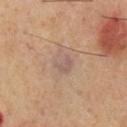| key | value |
|---|---|
| follow-up | imaged on a skin check; not biopsied |
| anatomic site | the chest |
| TBP lesion metrics | a footprint of about 4.5 mm², a shape eccentricity near 0.4, and a shape-asymmetry score of about 0.2 (0 = symmetric) |
| subject | male, roughly 70 years of age |
| lesion diameter | about 2.5 mm |
| image source | ~15 mm crop, total-body skin-cancer survey |